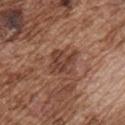biopsy_status: not biopsied; imaged during a skin examination
site: front of the torso
patient:
  sex: male
  age_approx: 75
image:
  source: total-body photography crop
  field_of_view_mm: 15